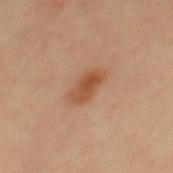Part of a total-body skin-imaging series; this lesion was reviewed on a skin check and was not flagged for biopsy.
The patient is a male in their 60s.
On the mid back.
A 15 mm close-up extracted from a 3D total-body photography capture.